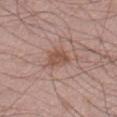| feature | finding |
|---|---|
| notes | catalogued during a skin exam; not biopsied |
| site | the right thigh |
| lesion diameter | ~3 mm (longest diameter) |
| image source | 15 mm crop, total-body photography |
| patient | male, aged approximately 60 |
| image-analysis metrics | an outline eccentricity of about 0.65 (0 = round, 1 = elongated) and a symmetry-axis asymmetry near 0.2; border irregularity of about 2.5 on a 0–10 scale, internal color variation of about 2.5 on a 0–10 scale, and a peripheral color-asymmetry measure near 1 |
| illumination | white-light |